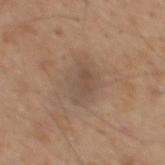Captured during whole-body skin photography for melanoma surveillance; the lesion was not biopsied.
The lesion-visualizer software estimated a footprint of about 10 mm², an eccentricity of roughly 0.7, and two-axis asymmetry of about 0.2. It also reported a border-irregularity index near 2.5/10.
On the mid back.
This is a white-light tile.
Cropped from a whole-body photographic skin survey; the tile spans about 15 mm.
The patient is a male in their mid-40s.
The lesion's longest dimension is about 4.5 mm.